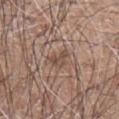workup: no biopsy performed (imaged during a skin exam)
illumination: white-light
patient: male, in their 60s
body site: the left forearm
diameter: ~2.5 mm (longest diameter)
acquisition: ~15 mm crop, total-body skin-cancer survey